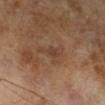follow-up: no biopsy performed (imaged during a skin exam); image source: ~15 mm tile from a whole-body skin photo; patient: male, in their mid-60s.